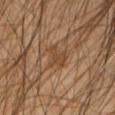Part of a total-body skin-imaging series; this lesion was reviewed on a skin check and was not flagged for biopsy. Longest diameter approximately 3 mm. A male subject in their mid- to late 40s. A 15 mm crop from a total-body photograph taken for skin-cancer surveillance. Automated tile analysis of the lesion measured an outline eccentricity of about 0.4 (0 = round, 1 = elongated) and a shape-asymmetry score of about 0.45 (0 = symmetric). And it measured internal color variation of about 1 on a 0–10 scale and radial color variation of about 0.5. The lesion is on the left forearm. Captured under cross-polarized illumination.